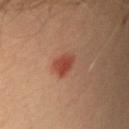{"biopsy_status": "not biopsied; imaged during a skin examination", "image": {"source": "total-body photography crop", "field_of_view_mm": 15}, "patient": {"sex": "male", "age_approx": 40}, "lesion_size": {"long_diameter_mm_approx": 3.0}, "automated_metrics": {"cielab_L": 45, "cielab_a": 29, "cielab_b": 32, "vs_skin_darker_L": 10.0, "vs_skin_contrast_norm": 8.0, "color_variation_0_10": 2.0, "lesion_detection_confidence_0_100": 100}, "lighting": "cross-polarized", "site": "left upper arm"}